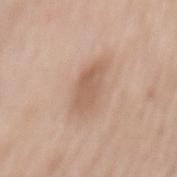Case summary:
- notes · catalogued during a skin exam; not biopsied
- patient · female, in their mid- to late 70s
- diameter · ~5 mm (longest diameter)
- location · the mid back
- imaging modality · ~15 mm tile from a whole-body skin photo
- tile lighting · white-light illumination